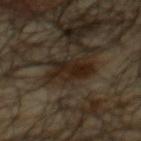The lesion was photographed on a routine skin check and not biopsied; there is no pathology result.
A roughly 15 mm field-of-view crop from a total-body skin photograph.
An algorithmic analysis of the crop reported roughly 9 lightness units darker than nearby skin and a normalized border contrast of about 10.5. The software also gave border irregularity of about 4.5 on a 0–10 scale and a color-variation rating of about 3.5/10.
A male patient aged 63–67.
Captured under cross-polarized illumination.
Measured at roughly 4.5 mm in maximum diameter.
The lesion is located on the mid back.